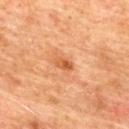Findings:
• workup — total-body-photography surveillance lesion; no biopsy
• illumination — cross-polarized illumination
• lesion size — ~2.5 mm (longest diameter)
• body site — the mid back
• subject — male, aged 73–77
• image — 15 mm crop, total-body photography